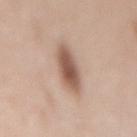This lesion was catalogued during total-body skin photography and was not selected for biopsy. The lesion is on the mid back. A female patient aged approximately 50. The lesion's longest dimension is about 4.5 mm. This image is a 15 mm lesion crop taken from a total-body photograph. Automated image analysis of the tile measured a lesion area of about 9.5 mm² and an outline eccentricity of about 0.85 (0 = round, 1 = elongated). The analysis additionally found a classifier nevus-likeness of about 95/100. Imaged with white-light lighting.